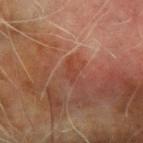Q: Was this lesion biopsied?
A: no biopsy performed (imaged during a skin exam)
Q: Patient demographics?
A: male, roughly 70 years of age
Q: Where on the body is the lesion?
A: the left forearm
Q: What kind of image is this?
A: ~15 mm tile from a whole-body skin photo
Q: How was the tile lit?
A: cross-polarized illumination